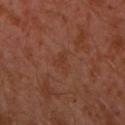Recorded during total-body skin imaging; not selected for excision or biopsy.
A 15 mm crop from a total-body photograph taken for skin-cancer surveillance.
The patient is a male in their 30s.
The lesion's longest dimension is about 3 mm.
Located on the left upper arm.
The tile uses cross-polarized illumination.